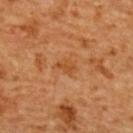notes=total-body-photography surveillance lesion; no biopsy | image=~15 mm tile from a whole-body skin photo | subject=female, about 55 years old | site=the upper back.